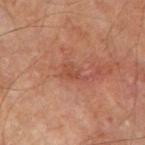Imaged during a routine full-body skin examination; the lesion was not biopsied and no histopathology is available. From the right upper arm. A lesion tile, about 15 mm wide, cut from a 3D total-body photograph. A male patient in their mid- to late 60s.